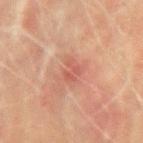Recorded during total-body skin imaging; not selected for excision or biopsy. On the left upper arm. A male subject, aged around 70. Captured under cross-polarized illumination. The recorded lesion diameter is about 2.5 mm. A lesion tile, about 15 mm wide, cut from a 3D total-body photograph.